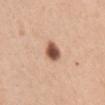Notes:
- workup: imaged on a skin check; not biopsied
- automated lesion analysis: a footprint of about 5.5 mm² and a symmetry-axis asymmetry near 0.25
- lighting: white-light illumination
- patient: female, roughly 30 years of age
- image: ~15 mm crop, total-body skin-cancer survey
- anatomic site: the chest